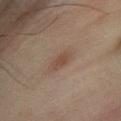Impression: No biopsy was performed on this lesion — it was imaged during a full skin examination and was not determined to be concerning. Image and clinical context: On the right lower leg. A 15 mm close-up extracted from a 3D total-body photography capture. The total-body-photography lesion software estimated about 8 CIELAB-L* units darker than the surrounding skin and a normalized lesion–skin contrast near 6.5. The subject is a male approximately 50 years of age. This is a cross-polarized tile.